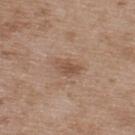{
  "biopsy_status": "not biopsied; imaged during a skin examination",
  "lighting": "white-light",
  "site": "upper back",
  "image": {
    "source": "total-body photography crop",
    "field_of_view_mm": 15
  },
  "patient": {
    "sex": "male",
    "age_approx": 50
  },
  "automated_metrics": {
    "border_irregularity_0_10": 3.0,
    "color_variation_0_10": 3.0,
    "peripheral_color_asymmetry": 1.0
  }
}